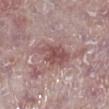Imaged during a routine full-body skin examination; the lesion was not biopsied and no histopathology is available. From the right lower leg. Cropped from a total-body skin-imaging series; the visible field is about 15 mm. This is a white-light tile. Approximately 4.5 mm at its widest. A male subject, aged around 75.